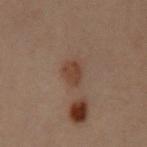{
  "biopsy_status": "not biopsied; imaged during a skin examination",
  "patient": {
    "sex": "female",
    "age_approx": 30
  },
  "lesion_size": {
    "long_diameter_mm_approx": 3.0
  },
  "site": "left upper arm",
  "lighting": "cross-polarized",
  "automated_metrics": {
    "eccentricity": 0.75,
    "cielab_L": 32,
    "cielab_a": 15,
    "cielab_b": 22,
    "vs_skin_darker_L": 7.0,
    "vs_skin_contrast_norm": 7.5,
    "nevus_likeness_0_100": 20,
    "lesion_detection_confidence_0_100": 100
  },
  "image": {
    "source": "total-body photography crop",
    "field_of_view_mm": 15
  }
}